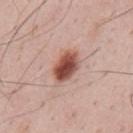{
  "biopsy_status": "not biopsied; imaged during a skin examination",
  "lesion_size": {
    "long_diameter_mm_approx": 4.0
  },
  "image": {
    "source": "total-body photography crop",
    "field_of_view_mm": 15
  },
  "site": "back",
  "patient": {
    "sex": "male",
    "age_approx": 35
  }
}